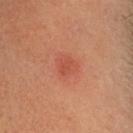Q: Was a biopsy performed?
A: total-body-photography surveillance lesion; no biopsy
Q: What is the lesion's diameter?
A: ~3 mm (longest diameter)
Q: Lesion location?
A: the head or neck
Q: What did automated image analysis measure?
A: a lesion area of about 6.5 mm² and a shape eccentricity near 0.4; an automated nevus-likeness rating near 15 out of 100
Q: Patient demographics?
A: male, roughly 45 years of age
Q: How was this image acquired?
A: ~15 mm crop, total-body skin-cancer survey